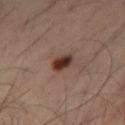Notes:
- biopsy status: catalogued during a skin exam; not biopsied
- imaging modality: 15 mm crop, total-body photography
- subject: male, about 50 years old
- lesion size: ~3 mm (longest diameter)
- site: the abdomen
- tile lighting: cross-polarized